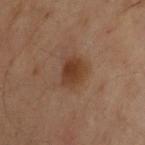Clinical impression:
No biopsy was performed on this lesion — it was imaged during a full skin examination and was not determined to be concerning.
Acquisition and patient details:
Cropped from a total-body skin-imaging series; the visible field is about 15 mm. The lesion is on the upper back. The patient is a male about 30 years old.